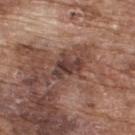Impression:
No biopsy was performed on this lesion — it was imaged during a full skin examination and was not determined to be concerning.
Clinical summary:
The recorded lesion diameter is about 4 mm. The lesion-visualizer software estimated border irregularity of about 4.5 on a 0–10 scale and a within-lesion color-variation index near 4.5/10. The tile uses white-light illumination. From the upper back. A male subject aged approximately 75. A 15 mm close-up extracted from a 3D total-body photography capture.